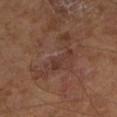Case summary:
• workup: catalogued during a skin exam; not biopsied
• image: total-body-photography crop, ~15 mm field of view
• lesion diameter: about 7.5 mm
• patient: male, in their mid-60s
• tile lighting: cross-polarized illumination